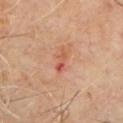Clinical impression:
Captured during whole-body skin photography for melanoma surveillance; the lesion was not biopsied.
Context:
A close-up tile cropped from a whole-body skin photograph, about 15 mm across. A male patient, aged 58 to 62. On the mid back.